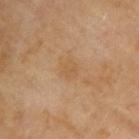Case summary:
• image source: total-body-photography crop, ~15 mm field of view
• patient: male, roughly 70 years of age
• lesion diameter: ~2.5 mm (longest diameter)
• lighting: cross-polarized illumination
• site: the right upper arm